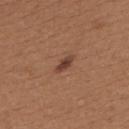Notes:
* workup: catalogued during a skin exam; not biopsied
* location: the back
* subject: female, aged approximately 40
* tile lighting: white-light illumination
* lesion diameter: ≈2.5 mm
* image: ~15 mm crop, total-body skin-cancer survey